Located on the right thigh; the patient is a female aged around 50; a 15 mm close-up extracted from a 3D total-body photography capture: 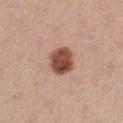Notes:
– lesion size: ~3.5 mm (longest diameter)
– illumination: white-light illumination
– histopathologic diagnosis: a dysplastic (Clark) nevus